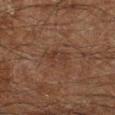biopsy status = total-body-photography surveillance lesion; no biopsy
subject = male, aged around 60
location = the right lower leg
acquisition = total-body-photography crop, ~15 mm field of view